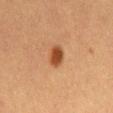Q: Automated lesion metrics?
A: an area of roughly 4.5 mm² and two-axis asymmetry of about 0.2; about 14 CIELAB-L* units darker than the surrounding skin and a normalized lesion–skin contrast near 10.5; a nevus-likeness score of about 100/100
Q: How was the tile lit?
A: cross-polarized illumination
Q: Where on the body is the lesion?
A: the abdomen
Q: How was this image acquired?
A: ~15 mm tile from a whole-body skin photo
Q: Who is the patient?
A: male, aged approximately 60